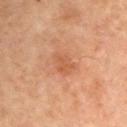Clinical impression: No biopsy was performed on this lesion — it was imaged during a full skin examination and was not determined to be concerning. Clinical summary: A male subject, aged approximately 70. An algorithmic analysis of the crop reported an average lesion color of about L≈43 a*≈20 b*≈29 (CIELAB). It also reported lesion-presence confidence of about 100/100. On the left upper arm. A region of skin cropped from a whole-body photographic capture, roughly 15 mm wide. Captured under cross-polarized illumination.